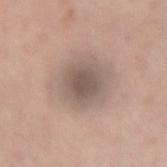No biopsy was performed on this lesion — it was imaged during a full skin examination and was not determined to be concerning.
Cropped from a total-body skin-imaging series; the visible field is about 15 mm.
A female subject, roughly 40 years of age.
The tile uses white-light illumination.
From the arm.
The recorded lesion diameter is about 4 mm.